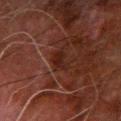This lesion was catalogued during total-body skin photography and was not selected for biopsy.
About 3 mm across.
The tile uses cross-polarized illumination.
Located on the arm.
A close-up tile cropped from a whole-body skin photograph, about 15 mm across.
A male patient aged around 80.
An algorithmic analysis of the crop reported a footprint of about 3.5 mm², an eccentricity of roughly 0.85, and two-axis asymmetry of about 0.45. The analysis additionally found a mean CIELAB color near L≈17 a*≈18 b*≈19, roughly 6 lightness units darker than nearby skin, and a normalized border contrast of about 7.5.